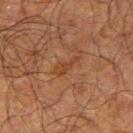Clinical impression:
The lesion was tiled from a total-body skin photograph and was not biopsied.
Acquisition and patient details:
About 3 mm across. The patient is a male roughly 70 years of age. Cropped from a whole-body photographic skin survey; the tile spans about 15 mm. The lesion is on the right upper arm. Captured under cross-polarized illumination.